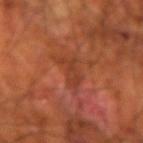Impression:
The lesion was photographed on a routine skin check and not biopsied; there is no pathology result.
Acquisition and patient details:
A male patient aged 68 to 72. From the left forearm. A region of skin cropped from a whole-body photographic capture, roughly 15 mm wide.